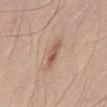Case summary:
– notes: total-body-photography surveillance lesion; no biopsy